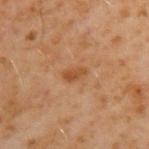Findings:
– follow-up: imaged on a skin check; not biopsied
– size: ≈2.5 mm
– image: ~15 mm tile from a whole-body skin photo
– body site: the upper back
– tile lighting: cross-polarized
– subject: male, roughly 60 years of age